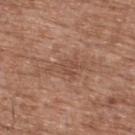{
  "biopsy_status": "not biopsied; imaged during a skin examination",
  "lighting": "white-light",
  "automated_metrics": {
    "area_mm2_approx": 6.5,
    "eccentricity": 0.9,
    "shape_asymmetry": 0.45,
    "vs_skin_contrast_norm": 5.0,
    "border_irregularity_0_10": 6.0,
    "color_variation_0_10": 2.0,
    "peripheral_color_asymmetry": 0.5,
    "nevus_likeness_0_100": 0
  },
  "image": {
    "source": "total-body photography crop",
    "field_of_view_mm": 15
  },
  "patient": {
    "sex": "male",
    "age_approx": 75
  },
  "lesion_size": {
    "long_diameter_mm_approx": 4.5
  },
  "site": "upper back"
}